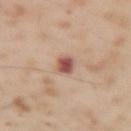imaging modality: ~15 mm tile from a whole-body skin photo; tile lighting: cross-polarized; patient: male, in their mid-50s; diameter: ≈2.5 mm; body site: the left upper arm.